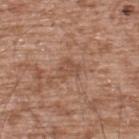follow-up: no biopsy performed (imaged during a skin exam) | subject: male, in their mid- to late 50s | diameter: about 2.5 mm | automated metrics: a lesion area of about 4.5 mm², an outline eccentricity of about 0.5 (0 = round, 1 = elongated), and two-axis asymmetry of about 0.35; an average lesion color of about L≈50 a*≈20 b*≈30 (CIELAB), roughly 7 lightness units darker than nearby skin, and a lesion-to-skin contrast of about 5.5 (normalized; higher = more distinct); border irregularity of about 3.5 on a 0–10 scale, internal color variation of about 1.5 on a 0–10 scale, and radial color variation of about 0.5; a nevus-likeness score of about 0/100 | image: total-body-photography crop, ~15 mm field of view | anatomic site: the upper back | lighting: white-light.